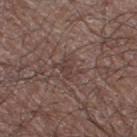Context: From the right thigh. The patient is a male aged 63–67. A lesion tile, about 15 mm wide, cut from a 3D total-body photograph.